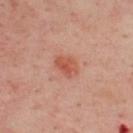Part of a total-body skin-imaging series; this lesion was reviewed on a skin check and was not flagged for biopsy.
A close-up tile cropped from a whole-body skin photograph, about 15 mm across.
From the upper back.
Imaged with cross-polarized lighting.
A male subject, aged around 50.
Longest diameter approximately 3 mm.